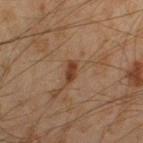workup: catalogued during a skin exam; not biopsied
patient: male, aged approximately 45
body site: the leg
image source: ~15 mm tile from a whole-body skin photo